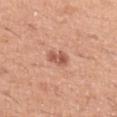Part of a total-body skin-imaging series; this lesion was reviewed on a skin check and was not flagged for biopsy. This image is a 15 mm lesion crop taken from a total-body photograph. Located on the right upper arm. The subject is a male in their 40s. About 2.5 mm across.